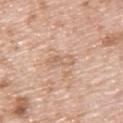Assessment:
Recorded during total-body skin imaging; not selected for excision or biopsy.
Acquisition and patient details:
A region of skin cropped from a whole-body photographic capture, roughly 15 mm wide. Automated image analysis of the tile measured an area of roughly 4.5 mm², a shape eccentricity near 0.95, and two-axis asymmetry of about 0.3. It also reported a border-irregularity index near 4/10. From the upper back. A male subject, aged 48 to 52.